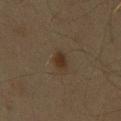| key | value |
|---|---|
| follow-up | imaged on a skin check; not biopsied |
| imaging modality | ~15 mm tile from a whole-body skin photo |
| body site | the front of the torso |
| illumination | cross-polarized |
| automated metrics | a lesion–skin lightness drop of about 7; a border-irregularity index near 2/10, a within-lesion color-variation index near 2/10, and peripheral color asymmetry of about 0.5 |
| size | about 2.5 mm |
| patient | male, aged 58 to 62 |